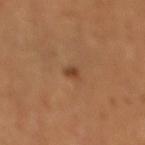biopsy_status: not biopsied; imaged during a skin examination
site: right lower leg
automated_metrics:
  eccentricity: 0.9
  shape_asymmetry: 0.3
  cielab_L: 43
  cielab_a: 21
  cielab_b: 34
  vs_skin_darker_L: 9.0
  vs_skin_contrast_norm: 7.5
  border_irregularity_0_10: 3.0
  color_variation_0_10: 0.0
  peripheral_color_asymmetry: 0.0
  nevus_likeness_0_100: 90
lighting: cross-polarized
lesion_size:
  long_diameter_mm_approx: 2.0
patient:
  sex: female
  age_approx: 55
image:
  source: total-body photography crop
  field_of_view_mm: 15